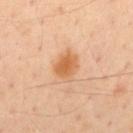Q: What is the imaging modality?
A: ~15 mm crop, total-body skin-cancer survey
Q: Where on the body is the lesion?
A: the mid back
Q: Patient demographics?
A: male, roughly 45 years of age
Q: What is the lesion's diameter?
A: ≈3.5 mm
Q: What lighting was used for the tile?
A: cross-polarized